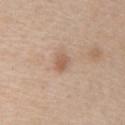| key | value |
|---|---|
| follow-up | catalogued during a skin exam; not biopsied |
| body site | the abdomen |
| subject | male, roughly 60 years of age |
| imaging modality | ~15 mm crop, total-body skin-cancer survey |
| lesion diameter | ~2.5 mm (longest diameter) |